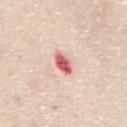Assessment: Captured during whole-body skin photography for melanoma surveillance; the lesion was not biopsied. Clinical summary: Cropped from a whole-body photographic skin survey; the tile spans about 15 mm. A male subject aged 78 to 82. The lesion is located on the mid back. Imaged with white-light lighting. Approximately 2.5 mm at its widest. The total-body-photography lesion software estimated a border-irregularity index near 1.5/10, a within-lesion color-variation index near 5.5/10, and peripheral color asymmetry of about 1.5. It also reported an automated nevus-likeness rating near 0 out of 100.